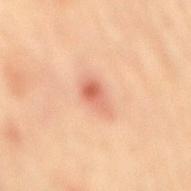<tbp_lesion>
<biopsy_status>not biopsied; imaged during a skin examination</biopsy_status>
<patient>
  <sex>female</sex>
  <age_approx>65</age_approx>
</patient>
<image>
  <source>total-body photography crop</source>
  <field_of_view_mm>15</field_of_view_mm>
</image>
<site>mid back</site>
</tbp_lesion>